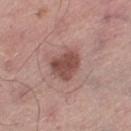notes: total-body-photography surveillance lesion; no biopsy | lighting: white-light illumination | body site: the left thigh | lesion diameter: ~3.5 mm (longest diameter) | patient: male, in their 70s | image: ~15 mm crop, total-body skin-cancer survey.